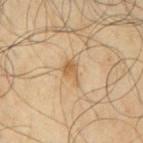{
  "patient": {
    "sex": "male",
    "age_approx": 65
  },
  "image": {
    "source": "total-body photography crop",
    "field_of_view_mm": 15
  },
  "automated_metrics": {
    "area_mm2_approx": 4.0,
    "eccentricity": 0.8,
    "cielab_L": 56,
    "cielab_a": 16,
    "cielab_b": 37,
    "vs_skin_darker_L": 9.0,
    "vs_skin_contrast_norm": 7.5,
    "nevus_likeness_0_100": 35
  },
  "lesion_size": {
    "long_diameter_mm_approx": 3.0
  },
  "lighting": "cross-polarized",
  "site": "mid back"
}